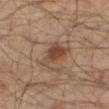Clinical impression: Part of a total-body skin-imaging series; this lesion was reviewed on a skin check and was not flagged for biopsy. Image and clinical context: From the right thigh. The patient is a male roughly 70 years of age. This image is a 15 mm lesion crop taken from a total-body photograph.